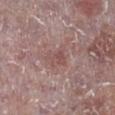| key | value |
|---|---|
| notes | total-body-photography surveillance lesion; no biopsy |
| patient | male, about 75 years old |
| site | the right lower leg |
| imaging modality | total-body-photography crop, ~15 mm field of view |
| automated lesion analysis | a footprint of about 6 mm² and an outline eccentricity of about 0.7 (0 = round, 1 = elongated); a border-irregularity index near 4/10, a color-variation rating of about 3/10, and peripheral color asymmetry of about 1; lesion-presence confidence of about 100/100 |
| tile lighting | white-light illumination |